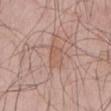| feature | finding |
|---|---|
| biopsy status | catalogued during a skin exam; not biopsied |
| subject | male, aged around 45 |
| automated metrics | an area of roughly 5 mm², a shape eccentricity near 0.7, and a shape-asymmetry score of about 0.35 (0 = symmetric); a mean CIELAB color near L≈57 a*≈20 b*≈28, about 5 CIELAB-L* units darker than the surrounding skin, and a normalized border contrast of about 5; a classifier nevus-likeness of about 0/100 and a detector confidence of about 95 out of 100 that the crop contains a lesion |
| lesion diameter | about 3 mm |
| tile lighting | white-light |
| acquisition | ~15 mm tile from a whole-body skin photo |
| anatomic site | the back |